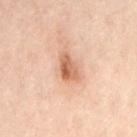notes: imaged on a skin check; not biopsied.